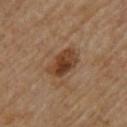biopsy status: imaged on a skin check; not biopsied | body site: the back | subject: male, roughly 85 years of age or older | image: ~15 mm tile from a whole-body skin photo | automated metrics: an automated nevus-likeness rating near 85 out of 100 and lesion-presence confidence of about 100/100 | tile lighting: cross-polarized illumination.